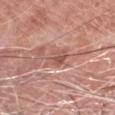workup=imaged on a skin check; not biopsied | tile lighting=white-light | image source=~15 mm tile from a whole-body skin photo | anatomic site=the head or neck | lesion diameter=about 2.5 mm | subject=male, in their 80s.